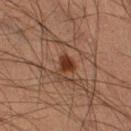biopsy status = total-body-photography surveillance lesion; no biopsy | imaging modality = total-body-photography crop, ~15 mm field of view | lesion size = ~3 mm (longest diameter) | lighting = cross-polarized | automated lesion analysis = two-axis asymmetry of about 0.5; an average lesion color of about L≈33 a*≈21 b*≈28 (CIELAB) and a normalized lesion–skin contrast near 10 | site = the right lower leg | patient = male, aged approximately 60.